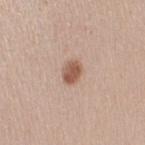Imaged during a routine full-body skin examination; the lesion was not biopsied and no histopathology is available. This is a white-light tile. A female patient aged around 40. Located on the right upper arm. This image is a 15 mm lesion crop taken from a total-body photograph. The lesion-visualizer software estimated an area of roughly 4.5 mm², an outline eccentricity of about 0.7 (0 = round, 1 = elongated), and two-axis asymmetry of about 0.15. Approximately 2.5 mm at its widest.